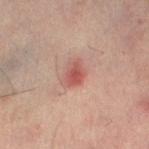Recorded during total-body skin imaging; not selected for excision or biopsy.
The lesion's longest dimension is about 3 mm.
A male patient roughly 50 years of age.
This is a cross-polarized tile.
Automated image analysis of the tile measured a lesion area of about 4.5 mm² and two-axis asymmetry of about 0.35. It also reported a border-irregularity index near 3/10, a color-variation rating of about 2/10, and a peripheral color-asymmetry measure near 0.5.
The lesion is on the left lower leg.
Cropped from a total-body skin-imaging series; the visible field is about 15 mm.